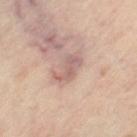Background:
A region of skin cropped from a whole-body photographic capture, roughly 15 mm wide. The tile uses cross-polarized illumination. Measured at roughly 4 mm in maximum diameter. A female subject, roughly 50 years of age. From the left thigh.